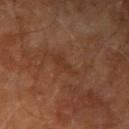| key | value |
|---|---|
| notes | total-body-photography surveillance lesion; no biopsy |
| size | ≈3.5 mm |
| location | the right upper arm |
| lighting | cross-polarized |
| image source | ~15 mm tile from a whole-body skin photo |
| subject | male, aged around 70 |